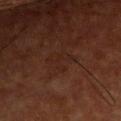Clinical impression: The lesion was tiled from a total-body skin photograph and was not biopsied. Image and clinical context: A male subject aged around 60. The lesion is on the chest. A region of skin cropped from a whole-body photographic capture, roughly 15 mm wide. The tile uses cross-polarized illumination. Automated image analysis of the tile measured a shape eccentricity near 0.75 and a shape-asymmetry score of about 0.4 (0 = symmetric). It also reported an average lesion color of about L≈21 a*≈18 b*≈22 (CIELAB).